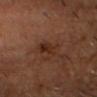{
  "biopsy_status": "not biopsied; imaged during a skin examination",
  "automated_metrics": {
    "area_mm2_approx": 5.5,
    "border_irregularity_0_10": 4.5,
    "color_variation_0_10": 4.0,
    "peripheral_color_asymmetry": 1.5
  },
  "patient": {
    "sex": "male",
    "age_approx": 60
  },
  "lighting": "cross-polarized",
  "site": "head or neck",
  "lesion_size": {
    "long_diameter_mm_approx": 3.5
  },
  "image": {
    "source": "total-body photography crop",
    "field_of_view_mm": 15
  }
}